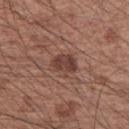| key | value |
|---|---|
| notes | imaged on a skin check; not biopsied |
| lesion diameter | about 3 mm |
| image source | total-body-photography crop, ~15 mm field of view |
| tile lighting | white-light illumination |
| image-analysis metrics | a footprint of about 6.5 mm², an outline eccentricity of about 0.55 (0 = round, 1 = elongated), and a symmetry-axis asymmetry near 0.2 |
| site | the right upper arm |
| subject | male, in their mid- to late 60s |